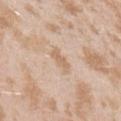follow-up: total-body-photography surveillance lesion; no biopsy | patient: female, aged around 25 | tile lighting: white-light illumination | imaging modality: 15 mm crop, total-body photography | body site: the left upper arm | lesion size: about 3 mm.